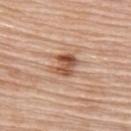No biopsy was performed on this lesion — it was imaged during a full skin examination and was not determined to be concerning. The patient is a female about 65 years old. The lesion is on the upper back. A lesion tile, about 15 mm wide, cut from a 3D total-body photograph. Imaged with white-light lighting. The total-body-photography lesion software estimated a footprint of about 6.5 mm², an eccentricity of roughly 0.6, and two-axis asymmetry of about 0.25. And it measured a classifier nevus-likeness of about 85/100 and a detector confidence of about 100 out of 100 that the crop contains a lesion.